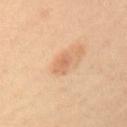Image and clinical context:
The lesion is on the upper back. Captured under cross-polarized illumination. Approximately 2.5 mm at its widest. A female patient, aged 33 to 37. A 15 mm crop from a total-body photograph taken for skin-cancer surveillance.